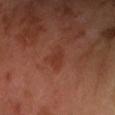Notes:
* workup — no biopsy performed (imaged during a skin exam)
* patient — male, in their mid-60s
* image source — ~15 mm crop, total-body skin-cancer survey
* lesion diameter — about 2.5 mm